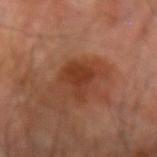A region of skin cropped from a whole-body photographic capture, roughly 15 mm wide.
An algorithmic analysis of the crop reported an area of roughly 7.5 mm², an outline eccentricity of about 0.55 (0 = round, 1 = elongated), and a shape-asymmetry score of about 0.4 (0 = symmetric). The analysis additionally found about 8 CIELAB-L* units darker than the surrounding skin and a lesion-to-skin contrast of about 7.5 (normalized; higher = more distinct).
A male patient, aged approximately 70.
The lesion's longest dimension is about 3.5 mm.
This is a cross-polarized tile.
On the right forearm.